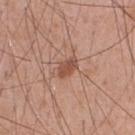Clinical impression: Recorded during total-body skin imaging; not selected for excision or biopsy. Acquisition and patient details: A roughly 15 mm field-of-view crop from a total-body skin photograph. This is a white-light tile. The lesion is on the chest. The lesion's longest dimension is about 3 mm. Automated image analysis of the tile measured border irregularity of about 2 on a 0–10 scale. The software also gave an automated nevus-likeness rating near 60 out of 100 and a lesion-detection confidence of about 100/100. The subject is a male aged around 55.